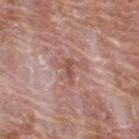No biopsy was performed on this lesion — it was imaged during a full skin examination and was not determined to be concerning.
A roughly 15 mm field-of-view crop from a total-body skin photograph.
Longest diameter approximately 2.5 mm.
On the upper back.
The patient is a female aged 58–62.